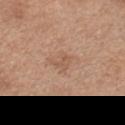Q: What is the imaging modality?
A: total-body-photography crop, ~15 mm field of view
Q: Lesion size?
A: ≈2.5 mm
Q: What are the patient's age and sex?
A: male, aged approximately 65
Q: Where on the body is the lesion?
A: the right upper arm
Q: What did automated image analysis measure?
A: a shape-asymmetry score of about 0.45 (0 = symmetric)